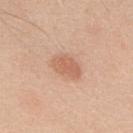Assessment: No biopsy was performed on this lesion — it was imaged during a full skin examination and was not determined to be concerning. Clinical summary: A male subject in their 50s. Cropped from a whole-body photographic skin survey; the tile spans about 15 mm. From the upper back. Approximately 3.5 mm at its widest.